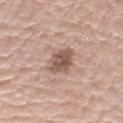<tbp_lesion>
<biopsy_status>not biopsied; imaged during a skin examination</biopsy_status>
<automated_metrics>
  <area_mm2_approx>8.0</area_mm2_approx>
  <eccentricity>0.75</eccentricity>
  <shape_asymmetry>0.2</shape_asymmetry>
  <cielab_L>55</cielab_L>
  <cielab_a>18</cielab_a>
  <cielab_b>25</cielab_b>
  <vs_skin_contrast_norm>8.5</vs_skin_contrast_norm>
  <border_irregularity_0_10>2.5</border_irregularity_0_10>
  <peripheral_color_asymmetry>1.0</peripheral_color_asymmetry>
</automated_metrics>
<lighting>white-light</lighting>
<site>left upper arm</site>
<lesion_size>
  <long_diameter_mm_approx>4.0</long_diameter_mm_approx>
</lesion_size>
<patient>
  <sex>male</sex>
  <age_approx>75</age_approx>
</patient>
<image>
  <source>total-body photography crop</source>
  <field_of_view_mm>15</field_of_view_mm>
</image>
</tbp_lesion>